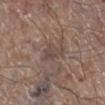* workup: catalogued during a skin exam; not biopsied
* patient: male, aged around 65
* location: the right lower leg
* acquisition: ~15 mm tile from a whole-body skin photo